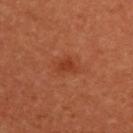notes: catalogued during a skin exam; not biopsied
image source: ~15 mm tile from a whole-body skin photo
diameter: ~2.5 mm (longest diameter)
patient: female, approximately 50 years of age